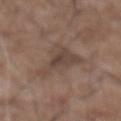This lesion was catalogued during total-body skin photography and was not selected for biopsy.